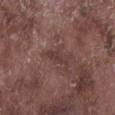Clinical impression:
Captured during whole-body skin photography for melanoma surveillance; the lesion was not biopsied.
Clinical summary:
A roughly 15 mm field-of-view crop from a total-body skin photograph. The lesion is on the left lower leg. The lesion's longest dimension is about 3.5 mm. This is a white-light tile. A male subject aged 73 to 77.